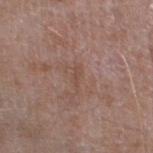Assessment: Imaged during a routine full-body skin examination; the lesion was not biopsied and no histopathology is available. Background: The tile uses white-light illumination. The recorded lesion diameter is about 3 mm. The patient is a male aged approximately 65. A lesion tile, about 15 mm wide, cut from a 3D total-body photograph. On the leg. The total-body-photography lesion software estimated roughly 5 lightness units darker than nearby skin and a normalized lesion–skin contrast near 4.5. And it measured a nevus-likeness score of about 0/100 and a lesion-detection confidence of about 100/100.